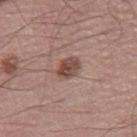Impression: Recorded during total-body skin imaging; not selected for excision or biopsy.